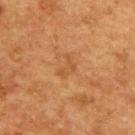Clinical impression: No biopsy was performed on this lesion — it was imaged during a full skin examination and was not determined to be concerning. Acquisition and patient details: Cropped from a total-body skin-imaging series; the visible field is about 15 mm. From the upper back. A male patient, about 75 years old.